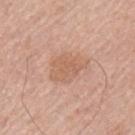{"biopsy_status": "not biopsied; imaged during a skin examination", "lighting": "white-light", "automated_metrics": {"border_irregularity_0_10": 3.0, "color_variation_0_10": 2.5, "peripheral_color_asymmetry": 1.0}, "patient": {"sex": "male", "age_approx": 55}, "image": {"source": "total-body photography crop", "field_of_view_mm": 15}, "lesion_size": {"long_diameter_mm_approx": 4.5}, "site": "arm"}